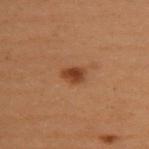No biopsy was performed on this lesion — it was imaged during a full skin examination and was not determined to be concerning. A 15 mm crop from a total-body photograph taken for skin-cancer surveillance. On the upper back. The patient is a female approximately 50 years of age.